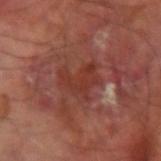This lesion was catalogued during total-body skin photography and was not selected for biopsy.